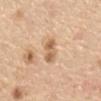follow-up: no biopsy performed (imaged during a skin exam)
lesion diameter: about 3.5 mm
patient: male, aged approximately 70
acquisition: 15 mm crop, total-body photography
illumination: white-light
anatomic site: the abdomen
automated lesion analysis: an outline eccentricity of about 0.85 (0 = round, 1 = elongated) and two-axis asymmetry of about 0.15; border irregularity of about 2 on a 0–10 scale, a color-variation rating of about 3/10, and peripheral color asymmetry of about 1; a detector confidence of about 100 out of 100 that the crop contains a lesion